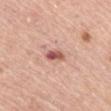Q: Was this lesion biopsied?
A: catalogued during a skin exam; not biopsied
Q: What lighting was used for the tile?
A: white-light illumination
Q: How was this image acquired?
A: total-body-photography crop, ~15 mm field of view
Q: Lesion size?
A: ≈2.5 mm
Q: Who is the patient?
A: female, roughly 65 years of age
Q: Lesion location?
A: the upper back
Q: What did automated image analysis measure?
A: a lesion area of about 3.5 mm² and a shape eccentricity near 0.85; a mean CIELAB color near L≈56 a*≈27 b*≈27 and roughly 15 lightness units darker than nearby skin; a border-irregularity index near 2/10 and peripheral color asymmetry of about 2; a nevus-likeness score of about 70/100 and a detector confidence of about 100 out of 100 that the crop contains a lesion